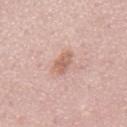Notes:
– follow-up — imaged on a skin check; not biopsied
– subject — male, aged 48–52
– lesion diameter — ≈3.5 mm
– site — the mid back
– image — ~15 mm tile from a whole-body skin photo